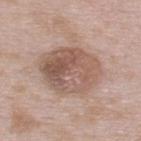biopsy_status: not biopsied; imaged during a skin examination
image:
  source: total-body photography crop
  field_of_view_mm: 15
lighting: white-light
lesion_size:
  long_diameter_mm_approx: 6.5
patient:
  sex: male
  age_approx: 55
automated_metrics:
  area_mm2_approx: 26.0
site: upper back